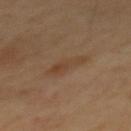Q: Where on the body is the lesion?
A: the mid back
Q: What are the patient's age and sex?
A: male, aged around 65
Q: How was this image acquired?
A: total-body-photography crop, ~15 mm field of view
Q: What lighting was used for the tile?
A: cross-polarized